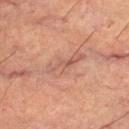The lesion was tiled from a total-body skin photograph and was not biopsied. Imaged with cross-polarized lighting. An algorithmic analysis of the crop reported a shape eccentricity near 0.9 and two-axis asymmetry of about 0.3. And it measured a mean CIELAB color near L≈54 a*≈22 b*≈27, roughly 6 lightness units darker than nearby skin, and a normalized lesion–skin contrast near 5. A male patient aged 58–62. About 4.5 mm across. The lesion is located on the left thigh. A 15 mm close-up extracted from a 3D total-body photography capture.